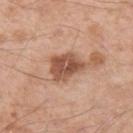  biopsy_status: not biopsied; imaged during a skin examination
  image:
    source: total-body photography crop
    field_of_view_mm: 15
  site: left upper arm
  automated_metrics:
    area_mm2_approx: 10.0
    eccentricity: 0.65
    shape_asymmetry: 0.35
    border_irregularity_0_10: 3.5
    color_variation_0_10: 4.5
    peripheral_color_asymmetry: 1.5
    nevus_likeness_0_100: 30
  patient:
    sex: male
    age_approx: 55
  lesion_size:
    long_diameter_mm_approx: 4.5